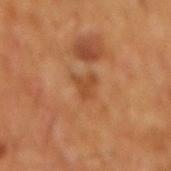Assessment: This lesion was catalogued during total-body skin photography and was not selected for biopsy. Context: The lesion is located on the abdomen. Automated image analysis of the tile measured a mean CIELAB color near L≈42 a*≈23 b*≈35, about 7 CIELAB-L* units darker than the surrounding skin, and a normalized lesion–skin contrast near 6. And it measured a color-variation rating of about 1/10 and a peripheral color-asymmetry measure near 0. A male patient roughly 60 years of age. This image is a 15 mm lesion crop taken from a total-body photograph.